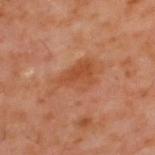tile lighting=cross-polarized illumination | imaging modality=15 mm crop, total-body photography | diameter=≈6.5 mm | subject=male, aged 58 to 62 | body site=the upper back.